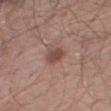<tbp_lesion>
  <biopsy_status>not biopsied; imaged during a skin examination</biopsy_status>
  <image>
    <source>total-body photography crop</source>
    <field_of_view_mm>15</field_of_view_mm>
  </image>
  <site>leg</site>
  <lighting>white-light</lighting>
  <patient>
    <sex>male</sex>
    <age_approx>70</age_approx>
  </patient>
</tbp_lesion>